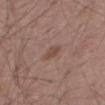notes = total-body-photography surveillance lesion; no biopsy | illumination = white-light | location = the leg | TBP lesion metrics = an area of roughly 3.5 mm², an eccentricity of roughly 0.85, and two-axis asymmetry of about 0.25; about 7 CIELAB-L* units darker than the surrounding skin and a normalized lesion–skin contrast near 6; an automated nevus-likeness rating near 0 out of 100 and a detector confidence of about 100 out of 100 that the crop contains a lesion | image source = 15 mm crop, total-body photography | patient = male, in their mid- to late 50s.